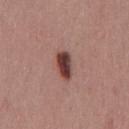Clinical impression:
The lesion was tiled from a total-body skin photograph and was not biopsied.
Context:
A close-up tile cropped from a whole-body skin photograph, about 15 mm across. Captured under white-light illumination. Automated image analysis of the tile measured a lesion–skin lightness drop of about 17 and a lesion-to-skin contrast of about 13 (normalized; higher = more distinct). The software also gave a border-irregularity index near 2/10, internal color variation of about 4.5 on a 0–10 scale, and radial color variation of about 1.5. The software also gave an automated nevus-likeness rating near 100 out of 100 and a detector confidence of about 100 out of 100 that the crop contains a lesion. A male subject, about 40 years old. Measured at roughly 3.5 mm in maximum diameter. The lesion is on the front of the torso.